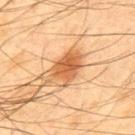Case summary:
– biopsy status · imaged on a skin check; not biopsied
– imaging modality · ~15 mm tile from a whole-body skin photo
– patient · male, in their mid- to late 40s
– site · the mid back